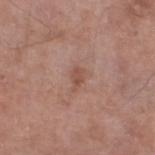Recorded during total-body skin imaging; not selected for excision or biopsy. The lesion-visualizer software estimated a lesion color around L≈51 a*≈21 b*≈26 in CIELAB, roughly 8 lightness units darker than nearby skin, and a normalized border contrast of about 6. The software also gave border irregularity of about 3.5 on a 0–10 scale and a color-variation rating of about 1/10. The lesion is located on the left thigh. The subject is a male aged approximately 80. The recorded lesion diameter is about 2.5 mm. A 15 mm close-up tile from a total-body photography series done for melanoma screening. Imaged with white-light lighting.